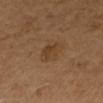biopsy status — catalogued during a skin exam; not biopsied
image source — 15 mm crop, total-body photography
lighting — cross-polarized illumination
lesion diameter — ≈3 mm
subject — female, in their mid-50s
site — the arm
automated lesion analysis — a footprint of about 6 mm², an eccentricity of roughly 0.55, and a shape-asymmetry score of about 0.25 (0 = symmetric); a lesion–skin lightness drop of about 6 and a lesion-to-skin contrast of about 6 (normalized; higher = more distinct); a border-irregularity rating of about 2.5/10, internal color variation of about 3 on a 0–10 scale, and peripheral color asymmetry of about 1; an automated nevus-likeness rating near 35 out of 100 and a detector confidence of about 100 out of 100 that the crop contains a lesion